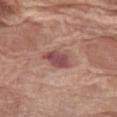notes: imaged on a skin check; not biopsied.